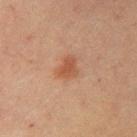workup = no biopsy performed (imaged during a skin exam) | acquisition = total-body-photography crop, ~15 mm field of view | anatomic site = the right upper arm | lighting = cross-polarized | patient = male, aged around 60.